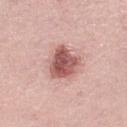follow-up: total-body-photography surveillance lesion; no biopsy
patient: female, aged approximately 65
TBP lesion metrics: a mean CIELAB color near L≈56 a*≈25 b*≈24, about 16 CIELAB-L* units darker than the surrounding skin, and a normalized border contrast of about 10; a detector confidence of about 100 out of 100 that the crop contains a lesion
acquisition: 15 mm crop, total-body photography
lighting: white-light
anatomic site: the left lower leg